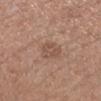<case>
<biopsy_status>not biopsied; imaged during a skin examination</biopsy_status>
<patient>
  <sex>female</sex>
  <age_approx>30</age_approx>
</patient>
<site>arm</site>
<automated_metrics>
  <area_mm2_approx>7.0</area_mm2_approx>
  <eccentricity>0.7</eccentricity>
  <shape_asymmetry>0.15</shape_asymmetry>
  <cielab_L>52</cielab_L>
  <cielab_a>18</cielab_a>
  <cielab_b>27</cielab_b>
  <vs_skin_darker_L>7.0</vs_skin_darker_L>
</automated_metrics>
<lesion_size>
  <long_diameter_mm_approx>3.5</long_diameter_mm_approx>
</lesion_size>
<image>
  <source>total-body photography crop</source>
  <field_of_view_mm>15</field_of_view_mm>
</image>
</case>